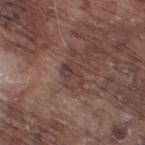  biopsy_status: not biopsied; imaged during a skin examination
  site: right thigh
  lighting: white-light
  image:
    source: total-body photography crop
    field_of_view_mm: 15
  lesion_size:
    long_diameter_mm_approx: 3.0
  patient:
    sex: male
    age_approx: 75